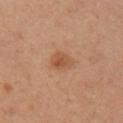Clinical impression: The lesion was photographed on a routine skin check and not biopsied; there is no pathology result. Context: The lesion is located on the arm. Cropped from a whole-body photographic skin survey; the tile spans about 15 mm. The lesion's longest dimension is about 2.5 mm. This is a cross-polarized tile. A male subject, aged 38 to 42.